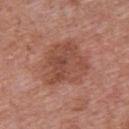The lesion-visualizer software estimated a mean CIELAB color near L≈48 a*≈24 b*≈28, a lesion–skin lightness drop of about 9, and a normalized lesion–skin contrast near 6.5. And it measured a border-irregularity rating of about 3/10 and peripheral color asymmetry of about 2. It also reported a classifier nevus-likeness of about 10/100 and a detector confidence of about 100 out of 100 that the crop contains a lesion. From the upper back. Approximately 6 mm at its widest. Cropped from a total-body skin-imaging series; the visible field is about 15 mm. A female patient, aged around 40.